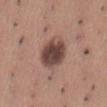| key | value |
|---|---|
| site | the abdomen |
| imaging modality | total-body-photography crop, ~15 mm field of view |
| diameter | about 4.5 mm |
| patient | male, aged around 45 |
| TBP lesion metrics | an outline eccentricity of about 0.6 (0 = round, 1 = elongated) and a symmetry-axis asymmetry near 0.1; roughly 15 lightness units darker than nearby skin and a lesion-to-skin contrast of about 11 (normalized; higher = more distinct); a within-lesion color-variation index near 5.5/10 and peripheral color asymmetry of about 1.5; a classifier nevus-likeness of about 30/100 and a detector confidence of about 100 out of 100 that the crop contains a lesion |